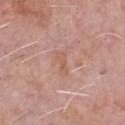notes = total-body-photography surveillance lesion; no biopsy.